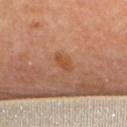Notes:
• workup · catalogued during a skin exam; not biopsied
• subject · female, roughly 60 years of age
• lesion size · ≈2.5 mm
• image-analysis metrics · a lesion area of about 3.5 mm², an eccentricity of roughly 0.8, and two-axis asymmetry of about 0.3; a lesion color around L≈52 a*≈25 b*≈38 in CIELAB and a lesion-to-skin contrast of about 6.5 (normalized; higher = more distinct)
• body site · the upper back
• image · total-body-photography crop, ~15 mm field of view
• illumination · cross-polarized